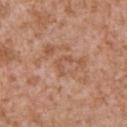Q: Was a biopsy performed?
A: no biopsy performed (imaged during a skin exam)
Q: What is the imaging modality?
A: total-body-photography crop, ~15 mm field of view
Q: Where on the body is the lesion?
A: the arm
Q: Patient demographics?
A: male, approximately 45 years of age
Q: What lighting was used for the tile?
A: white-light